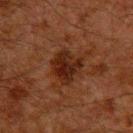Clinical impression:
The lesion was photographed on a routine skin check and not biopsied; there is no pathology result.
Clinical summary:
From the upper back. A roughly 15 mm field-of-view crop from a total-body skin photograph. The tile uses cross-polarized illumination. The patient is a male aged approximately 60.